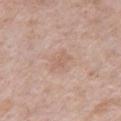workup=imaged on a skin check; not biopsied
subject=male, aged 48–52
location=the chest
acquisition=total-body-photography crop, ~15 mm field of view